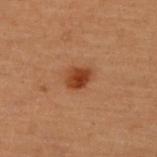workup — imaged on a skin check; not biopsied
lesion diameter — about 2.5 mm
site — the upper back
subject — female, aged around 50
acquisition — ~15 mm tile from a whole-body skin photo
automated metrics — a footprint of about 4.5 mm², a shape eccentricity near 0.65, and a symmetry-axis asymmetry near 0.15; roughly 11 lightness units darker than nearby skin and a lesion-to-skin contrast of about 10 (normalized; higher = more distinct); a nevus-likeness score of about 100/100